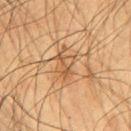biopsy_status: not biopsied; imaged during a skin examination
site: chest
patient:
  sex: male
  age_approx: 65
lighting: cross-polarized
image:
  source: total-body photography crop
  field_of_view_mm: 15